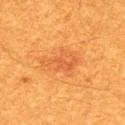follow-up: no biopsy performed (imaged during a skin exam); subject: male, in their 60s; automated metrics: a footprint of about 10 mm² and a symmetry-axis asymmetry near 0.35; site: the upper back; lesion size: ~4.5 mm (longest diameter); image source: ~15 mm tile from a whole-body skin photo; tile lighting: cross-polarized illumination.